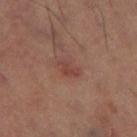biopsy status: total-body-photography surveillance lesion; no biopsy
tile lighting: cross-polarized
image source: 15 mm crop, total-body photography
TBP lesion metrics: an average lesion color of about L≈44 a*≈24 b*≈25 (CIELAB), roughly 7 lightness units darker than nearby skin, and a normalized lesion–skin contrast near 6; a border-irregularity index near 3/10, internal color variation of about 1.5 on a 0–10 scale, and a peripheral color-asymmetry measure near 0.5; a classifier nevus-likeness of about 0/100 and lesion-presence confidence of about 100/100
lesion diameter: about 3 mm
location: the left thigh